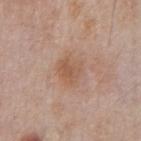tile lighting: white-light illumination
image: 15 mm crop, total-body photography
TBP lesion metrics: internal color variation of about 3 on a 0–10 scale and radial color variation of about 1
anatomic site: the chest
size: ~3.5 mm (longest diameter)
patient: male, aged approximately 80